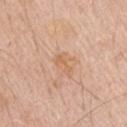  biopsy_status: not biopsied; imaged during a skin examination
  patient:
    sex: male
    age_approx: 60
  image:
    source: total-body photography crop
    field_of_view_mm: 15
  lesion_size:
    long_diameter_mm_approx: 3.0
  lighting: white-light
  site: right upper arm